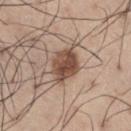notes=imaged on a skin check; not biopsied
subject=male, aged approximately 55
size=about 3.5 mm
image-analysis metrics=an average lesion color of about L≈48 a*≈18 b*≈27 (CIELAB), roughly 15 lightness units darker than nearby skin, and a normalized border contrast of about 10.5; a within-lesion color-variation index near 5/10 and radial color variation of about 2; an automated nevus-likeness rating near 95 out of 100 and a detector confidence of about 100 out of 100 that the crop contains a lesion
imaging modality=~15 mm tile from a whole-body skin photo
tile lighting=white-light
body site=the right thigh